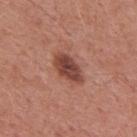{
  "biopsy_status": "not biopsied; imaged during a skin examination",
  "lesion_size": {
    "long_diameter_mm_approx": 4.5
  },
  "lighting": "white-light",
  "site": "mid back",
  "patient": {
    "sex": "male",
    "age_approx": 55
  },
  "image": {
    "source": "total-body photography crop",
    "field_of_view_mm": 15
  }
}